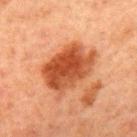The lesion was photographed on a routine skin check and not biopsied; there is no pathology result. Located on the chest. The lesion-visualizer software estimated a border-irregularity rating of about 2/10 and peripheral color asymmetry of about 1.5. And it measured lesion-presence confidence of about 100/100. Imaged with cross-polarized lighting. About 6.5 mm across. Cropped from a whole-body photographic skin survey; the tile spans about 15 mm. A male subject aged approximately 65.